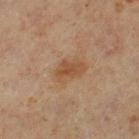notes: total-body-photography surveillance lesion; no biopsy
size: ~3.5 mm (longest diameter)
tile lighting: cross-polarized
subject: male, approximately 60 years of age
site: the left lower leg
acquisition: ~15 mm tile from a whole-body skin photo